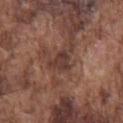workup = no biopsy performed (imaged during a skin exam) | imaging modality = 15 mm crop, total-body photography | patient = male, about 75 years old | anatomic site = the chest.